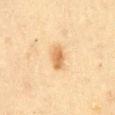Assessment:
Recorded during total-body skin imaging; not selected for excision or biopsy.
Acquisition and patient details:
A 15 mm crop from a total-body photograph taken for skin-cancer surveillance. The lesion's longest dimension is about 3 mm. The tile uses cross-polarized illumination. Automated image analysis of the tile measured an area of roughly 5 mm², a shape eccentricity near 0.8, and a shape-asymmetry score of about 0.15 (0 = symmetric). A female subject, aged 53–57. The lesion is located on the abdomen.